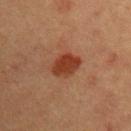workup — total-body-photography surveillance lesion; no biopsy
lesion size — ≈3.5 mm
patient — male, about 40 years old
location — the right upper arm
tile lighting — cross-polarized illumination
image — ~15 mm crop, total-body skin-cancer survey